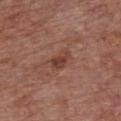Case summary:
• workup — no biopsy performed (imaged during a skin exam)
• lesion diameter — about 3 mm
• acquisition — 15 mm crop, total-body photography
• site — the chest
• tile lighting — white-light illumination
• patient — male, roughly 65 years of age